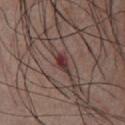Impression: Captured during whole-body skin photography for melanoma surveillance; the lesion was not biopsied. Background: The patient is a male aged around 55. The recorded lesion diameter is about 2.5 mm. A 15 mm crop from a total-body photograph taken for skin-cancer surveillance. Located on the chest.